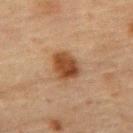- notes · catalogued during a skin exam; not biopsied
- illumination · cross-polarized illumination
- imaging modality · ~15 mm tile from a whole-body skin photo
- patient · male, aged around 75
- image-analysis metrics · a lesion area of about 8.5 mm², an eccentricity of roughly 0.6, and two-axis asymmetry of about 0.2; roughly 12 lightness units darker than nearby skin and a normalized border contrast of about 10.5; a within-lesion color-variation index near 3.5/10 and peripheral color asymmetry of about 1
- site · the back